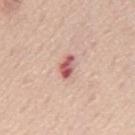workup: catalogued during a skin exam; not biopsied
image: ~15 mm crop, total-body skin-cancer survey
subject: male, in their 60s
anatomic site: the mid back